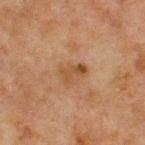• workup — imaged on a skin check; not biopsied
• tile lighting — cross-polarized
• patient — male, in their mid- to late 60s
• image source — 15 mm crop, total-body photography
• anatomic site — the front of the torso
• diameter — about 3 mm